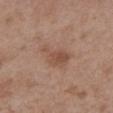Imaged during a routine full-body skin examination; the lesion was not biopsied and no histopathology is available. Imaged with white-light lighting. Located on the back. A male patient about 50 years old. The lesion's longest dimension is about 4.5 mm. A region of skin cropped from a whole-body photographic capture, roughly 15 mm wide.